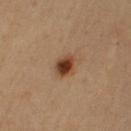Findings:
• size: about 3 mm
• patient: female, about 45 years old
• body site: the left upper arm
• lighting: cross-polarized illumination
• automated metrics: an area of roughly 5 mm², an outline eccentricity of about 0.55 (0 = round, 1 = elongated), and a shape-asymmetry score of about 0.2 (0 = symmetric)
• acquisition: ~15 mm tile from a whole-body skin photo